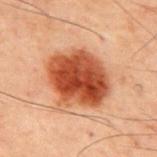{"site": "upper back", "patient": {"sex": "male", "age_approx": 60}, "image": {"source": "total-body photography crop", "field_of_view_mm": 15}}